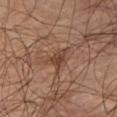Impression: Recorded during total-body skin imaging; not selected for excision or biopsy. Background: This is a cross-polarized tile. A 15 mm close-up extracted from a 3D total-body photography capture. On the right thigh. About 2.5 mm across. A male subject, about 65 years old.